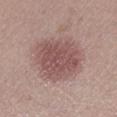Assessment:
Imaged during a routine full-body skin examination; the lesion was not biopsied and no histopathology is available.
Acquisition and patient details:
On the left lower leg. The recorded lesion diameter is about 5.5 mm. Automated tile analysis of the lesion measured a lesion area of about 25 mm², an eccentricity of roughly 0.25, and a symmetry-axis asymmetry near 0.15. The software also gave a classifier nevus-likeness of about 65/100. Captured under white-light illumination. A female patient aged approximately 20. A 15 mm close-up extracted from a 3D total-body photography capture.